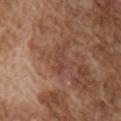The lesion was tiled from a total-body skin photograph and was not biopsied. Cropped from a whole-body photographic skin survey; the tile spans about 15 mm. The patient is a male aged 73–77. Automated image analysis of the tile measured a lesion area of about 3.5 mm², a shape eccentricity near 0.9, and a symmetry-axis asymmetry near 0.25. It also reported an average lesion color of about L≈43 a*≈22 b*≈26 (CIELAB), about 6 CIELAB-L* units darker than the surrounding skin, and a normalized lesion–skin contrast near 5.5. The software also gave a border-irregularity rating of about 3.5/10, internal color variation of about 0 on a 0–10 scale, and peripheral color asymmetry of about 0. It also reported a classifier nevus-likeness of about 0/100 and lesion-presence confidence of about 95/100. Measured at roughly 3.5 mm in maximum diameter. Captured under white-light illumination. The lesion is on the right upper arm.